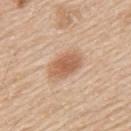Q: How was this image acquired?
A: total-body-photography crop, ~15 mm field of view
Q: Who is the patient?
A: male, aged approximately 60
Q: Where on the body is the lesion?
A: the upper back